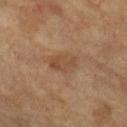Context: From the right lower leg. A female patient about 70 years old. A 15 mm crop from a total-body photograph taken for skin-cancer surveillance.